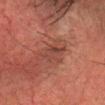The lesion is on the head or neck. The patient is a male aged 58 to 62. Longest diameter approximately 3 mm. This image is a 15 mm lesion crop taken from a total-body photograph. Imaged with cross-polarized lighting. Automated image analysis of the tile measured a mean CIELAB color near L≈32 a*≈20 b*≈22 and roughly 5 lightness units darker than nearby skin. The software also gave a border-irregularity rating of about 3/10, a color-variation rating of about 4/10, and a peripheral color-asymmetry measure near 1.5. It also reported an automated nevus-likeness rating near 5 out of 100.